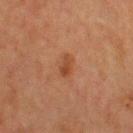{
  "biopsy_status": "not biopsied; imaged during a skin examination",
  "automated_metrics": {
    "eccentricity": 0.85,
    "shape_asymmetry": 0.3,
    "cielab_L": 38,
    "cielab_a": 22,
    "cielab_b": 30,
    "vs_skin_darker_L": 7.0
  },
  "lighting": "cross-polarized",
  "site": "upper back",
  "image": {
    "source": "total-body photography crop",
    "field_of_view_mm": 15
  },
  "lesion_size": {
    "long_diameter_mm_approx": 2.5
  },
  "patient": {
    "sex": "male",
    "age_approx": 60
  }
}